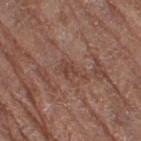Imaged during a routine full-body skin examination; the lesion was not biopsied and no histopathology is available. From the leg. This is a white-light tile. A 15 mm close-up extracted from a 3D total-body photography capture. A female patient aged around 80.